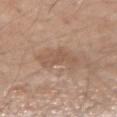Clinical impression:
Part of a total-body skin-imaging series; this lesion was reviewed on a skin check and was not flagged for biopsy.
Image and clinical context:
Cropped from a whole-body photographic skin survey; the tile spans about 15 mm. Imaged with white-light lighting. The lesion-visualizer software estimated a lesion area of about 8 mm² and an eccentricity of roughly 0.8. And it measured border irregularity of about 5 on a 0–10 scale, a color-variation rating of about 2/10, and peripheral color asymmetry of about 1. The patient is a male aged approximately 60. The lesion is located on the left forearm.